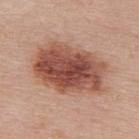Captured during whole-body skin photography for melanoma surveillance; the lesion was not biopsied.
Cropped from a whole-body photographic skin survey; the tile spans about 15 mm.
Imaged with white-light lighting.
The patient is a female roughly 50 years of age.
The recorded lesion diameter is about 8.5 mm.
Located on the upper back.